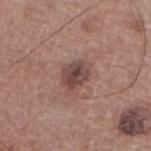Impression:
The lesion was tiled from a total-body skin photograph and was not biopsied.